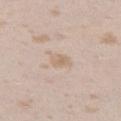Recorded during total-body skin imaging; not selected for excision or biopsy.
Captured under white-light illumination.
A female patient aged approximately 25.
The recorded lesion diameter is about 2.5 mm.
Automated tile analysis of the lesion measured a footprint of about 4 mm² and a shape-asymmetry score of about 0.2 (0 = symmetric). The analysis additionally found a lesion color around L≈66 a*≈14 b*≈29 in CIELAB and roughly 7 lightness units darker than nearby skin. It also reported a border-irregularity index near 2/10 and a peripheral color-asymmetry measure near 0.5.
A 15 mm close-up tile from a total-body photography series done for melanoma screening.
The lesion is on the left thigh.